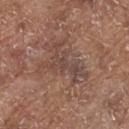Case summary:
- biopsy status — no biopsy performed (imaged during a skin exam)
- subject — male, aged approximately 80
- image source — ~15 mm tile from a whole-body skin photo
- site — the upper back